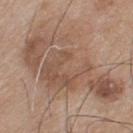Notes:
• biopsy status · total-body-photography surveillance lesion; no biopsy
• subject · male, aged 73 to 77
• anatomic site · the upper back
• acquisition · ~15 mm crop, total-body skin-cancer survey